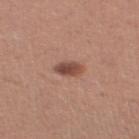| field | value |
|---|---|
| biopsy status | no biopsy performed (imaged during a skin exam) |
| lesion size | ~3 mm (longest diameter) |
| patient | female, in their 30s |
| automated metrics | a footprint of about 4.5 mm² and a symmetry-axis asymmetry near 0.15; a border-irregularity rating of about 1.5/10 and a color-variation rating of about 4.5/10 |
| illumination | white-light illumination |
| image source | total-body-photography crop, ~15 mm field of view |
| body site | the leg |